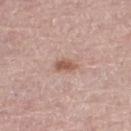Q: Was this lesion biopsied?
A: catalogued during a skin exam; not biopsied